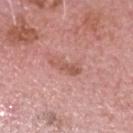• notes: total-body-photography surveillance lesion; no biopsy
• body site: the head or neck
• illumination: white-light
• size: ~3.5 mm (longest diameter)
• patient: male, aged 73 to 77
• acquisition: ~15 mm crop, total-body skin-cancer survey
• image-analysis metrics: an average lesion color of about L≈55 a*≈25 b*≈27 (CIELAB), a lesion–skin lightness drop of about 9, and a normalized lesion–skin contrast near 6.5; a border-irregularity index near 4/10, internal color variation of about 0.5 on a 0–10 scale, and peripheral color asymmetry of about 0.5